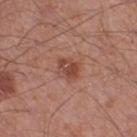<case>
<biopsy_status>not biopsied; imaged during a skin examination</biopsy_status>
<site>leg</site>
<image>
  <source>total-body photography crop</source>
  <field_of_view_mm>15</field_of_view_mm>
</image>
<lighting>white-light</lighting>
<patient>
  <sex>male</sex>
  <age_approx>55</age_approx>
</patient>
</case>